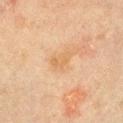notes = no biopsy performed (imaged during a skin exam) | illumination = cross-polarized | automated metrics = a footprint of about 3 mm²; a border-irregularity index near 5/10, a within-lesion color-variation index near 0.5/10, and radial color variation of about 0; an automated nevus-likeness rating near 0 out of 100 | image = 15 mm crop, total-body photography | patient = male, approximately 70 years of age | anatomic site = the left upper arm.